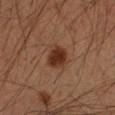| key | value |
|---|---|
| biopsy status | catalogued during a skin exam; not biopsied |
| location | the arm |
| lesion diameter | ~3 mm (longest diameter) |
| TBP lesion metrics | a footprint of about 6.5 mm², an outline eccentricity of about 0.5 (0 = round, 1 = elongated), and two-axis asymmetry of about 0.25; a border-irregularity rating of about 2/10 and internal color variation of about 2 on a 0–10 scale; an automated nevus-likeness rating near 100 out of 100 |
| image source | ~15 mm crop, total-body skin-cancer survey |
| subject | male, in their mid-30s |
| illumination | cross-polarized |